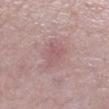biopsy_status: not biopsied; imaged during a skin examination
lesion_size:
  long_diameter_mm_approx: 3.0
image:
  source: total-body photography crop
  field_of_view_mm: 15
site: leg
lighting: white-light
automated_metrics:
  area_mm2_approx: 5.5
  eccentricity: 0.7
  nevus_likeness_0_100: 5
  lesion_detection_confidence_0_100: 100
patient:
  sex: female
  age_approx: 60